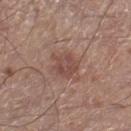biopsy status=catalogued during a skin exam; not biopsied | site=the right lower leg | image-analysis metrics=a within-lesion color-variation index near 2.5/10 and peripheral color asymmetry of about 1 | subject=male, roughly 55 years of age | image source=~15 mm crop, total-body skin-cancer survey.